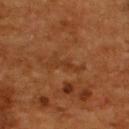<lesion>
  <biopsy_status>not biopsied; imaged during a skin examination</biopsy_status>
  <site>upper back</site>
  <patient>
    <sex>male</sex>
    <age_approx>55</age_approx>
  </patient>
  <lighting>cross-polarized</lighting>
  <image>
    <source>total-body photography crop</source>
    <field_of_view_mm>15</field_of_view_mm>
  </image>
</lesion>